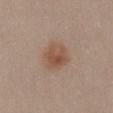Part of a total-body skin-imaging series; this lesion was reviewed on a skin check and was not flagged for biopsy. Approximately 3.5 mm at its widest. The subject is a female aged approximately 30. A lesion tile, about 15 mm wide, cut from a 3D total-body photograph. From the front of the torso. Imaged with white-light lighting.